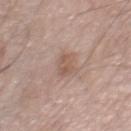biopsy status: imaged on a skin check; not biopsied | patient: male, aged around 80 | TBP lesion metrics: a shape eccentricity near 0.85; an automated nevus-likeness rating near 0 out of 100 | lesion size: about 3 mm | anatomic site: the right thigh | illumination: white-light illumination | image source: ~15 mm tile from a whole-body skin photo.